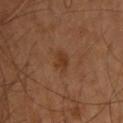notes: total-body-photography surveillance lesion; no biopsy | location: the upper back | image source: ~15 mm tile from a whole-body skin photo | subject: male, aged 58–62 | tile lighting: cross-polarized.